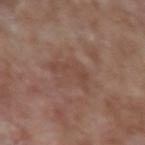follow-up: no biopsy performed (imaged during a skin exam); image source: ~15 mm tile from a whole-body skin photo; subject: male, approximately 65 years of age; site: the left upper arm; lesion diameter: ~4 mm (longest diameter); illumination: white-light.